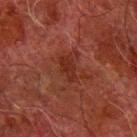No biopsy was performed on this lesion — it was imaged during a full skin examination and was not determined to be concerning.
The subject is a male aged 78 to 82.
From the arm.
Cropped from a whole-body photographic skin survey; the tile spans about 15 mm.
The recorded lesion diameter is about 3 mm.
Automated image analysis of the tile measured an area of roughly 4.5 mm², an eccentricity of roughly 0.85, and two-axis asymmetry of about 0.25. And it measured a classifier nevus-likeness of about 0/100 and a lesion-detection confidence of about 100/100.